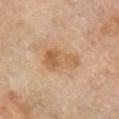{
  "biopsy_status": "not biopsied; imaged during a skin examination",
  "lighting": "cross-polarized",
  "image": {
    "source": "total-body photography crop",
    "field_of_view_mm": 15
  },
  "patient": {
    "sex": "male",
    "age_approx": 70
  },
  "site": "right upper arm"
}